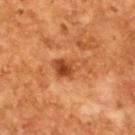| key | value |
|---|---|
| follow-up | no biopsy performed (imaged during a skin exam) |
| diameter | ~4 mm (longest diameter) |
| image source | ~15 mm crop, total-body skin-cancer survey |
| image-analysis metrics | border irregularity of about 4.5 on a 0–10 scale, a within-lesion color-variation index near 8/10, and radial color variation of about 3; an automated nevus-likeness rating near 20 out of 100 |
| subject | male, approximately 65 years of age |
| tile lighting | cross-polarized |